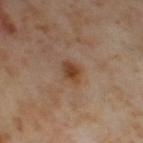<tbp_lesion>
  <biopsy_status>not biopsied; imaged during a skin examination</biopsy_status>
  <lighting>cross-polarized</lighting>
  <patient>
    <sex>female</sex>
    <age_approx>55</age_approx>
  </patient>
  <lesion_size>
    <long_diameter_mm_approx>3.0</long_diameter_mm_approx>
  </lesion_size>
  <site>right thigh</site>
  <image>
    <source>total-body photography crop</source>
    <field_of_view_mm>15</field_of_view_mm>
  </image>
</tbp_lesion>